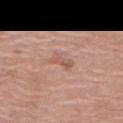– size: ≈3 mm
– patient: female, aged approximately 60
– image: ~15 mm crop, total-body skin-cancer survey
– body site: the left thigh
– TBP lesion metrics: a shape eccentricity near 0.9 and a symmetry-axis asymmetry near 0.25; an average lesion color of about L≈57 a*≈21 b*≈28 (CIELAB), about 7 CIELAB-L* units darker than the surrounding skin, and a normalized border contrast of about 5; border irregularity of about 3.5 on a 0–10 scale and a within-lesion color-variation index near 3/10; a classifier nevus-likeness of about 0/100
– lighting: white-light illumination